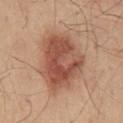Context: From the mid back. The lesion's longest dimension is about 7.5 mm. A 15 mm close-up tile from a total-body photography series done for melanoma screening. A male patient in their mid- to late 40s. Automated tile analysis of the lesion measured a lesion color around L≈52 a*≈23 b*≈30 in CIELAB and a lesion–skin lightness drop of about 12. And it measured an automated nevus-likeness rating near 100 out of 100.